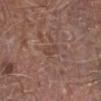Notes:
– biopsy status · no biopsy performed (imaged during a skin exam)
– image · ~15 mm tile from a whole-body skin photo
– subject · male, roughly 70 years of age
– body site · the right lower leg